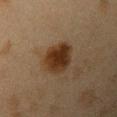Q: Was a biopsy performed?
A: no biopsy performed (imaged during a skin exam)
Q: Lesion location?
A: the left upper arm
Q: What lighting was used for the tile?
A: cross-polarized
Q: Patient demographics?
A: female, in their 40s
Q: How was this image acquired?
A: ~15 mm crop, total-body skin-cancer survey
Q: Lesion size?
A: about 4 mm